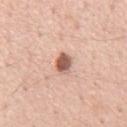Imaged during a routine full-body skin examination; the lesion was not biopsied and no histopathology is available. Automated image analysis of the tile measured a footprint of about 4.5 mm², a shape eccentricity near 0.75, and a symmetry-axis asymmetry near 0.25. It also reported a color-variation rating of about 4/10 and a peripheral color-asymmetry measure near 1. The analysis additionally found a nevus-likeness score of about 100/100 and lesion-presence confidence of about 100/100. A male patient roughly 55 years of age. A roughly 15 mm field-of-view crop from a total-body skin photograph. The tile uses white-light illumination. Measured at roughly 3 mm in maximum diameter. From the chest.